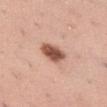{
  "biopsy_status": "not biopsied; imaged during a skin examination",
  "lesion_size": {
    "long_diameter_mm_approx": 3.5
  },
  "patient": {
    "sex": "female",
    "age_approx": 45
  },
  "site": "leg",
  "image": {
    "source": "total-body photography crop",
    "field_of_view_mm": 15
  }
}